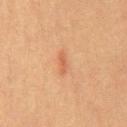| key | value |
|---|---|
| notes | catalogued during a skin exam; not biopsied |
| lesion diameter | about 3 mm |
| automated lesion analysis | a mean CIELAB color near L≈49 a*≈22 b*≈32, a lesion–skin lightness drop of about 7, and a normalized border contrast of about 5 |
| acquisition | ~15 mm tile from a whole-body skin photo |
| patient | male, in their 40s |
| anatomic site | the chest |